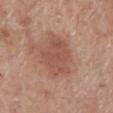Clinical impression:
The lesion was tiled from a total-body skin photograph and was not biopsied.
Background:
On the left lower leg. The recorded lesion diameter is about 4.5 mm. The tile uses white-light illumination. A region of skin cropped from a whole-body photographic capture, roughly 15 mm wide. Automated image analysis of the tile measured a lesion color around L≈52 a*≈24 b*≈28 in CIELAB, roughly 8 lightness units darker than nearby skin, and a lesion-to-skin contrast of about 5.5 (normalized; higher = more distinct). The software also gave a color-variation rating of about 2.5/10 and a peripheral color-asymmetry measure near 1. The patient is a male roughly 70 years of age.